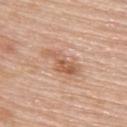Assessment: The lesion was tiled from a total-body skin photograph and was not biopsied. Context: Measured at roughly 5.5 mm in maximum diameter. Cropped from a whole-body photographic skin survey; the tile spans about 15 mm. The total-body-photography lesion software estimated a lesion area of about 10 mm², an outline eccentricity of about 0.9 (0 = round, 1 = elongated), and a symmetry-axis asymmetry near 0.3. From the upper back. A female subject roughly 65 years of age. The tile uses white-light illumination.